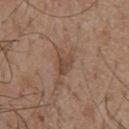Imaged during a routine full-body skin examination; the lesion was not biopsied and no histopathology is available.